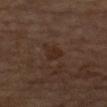Captured during whole-body skin photography for melanoma surveillance; the lesion was not biopsied.
Longest diameter approximately 2.5 mm.
A region of skin cropped from a whole-body photographic capture, roughly 15 mm wide.
Captured under cross-polarized illumination.
On the right forearm.
A female subject aged 68 to 72.